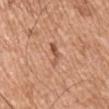Approximately 3 mm at its widest.
This image is a 15 mm lesion crop taken from a total-body photograph.
The patient is a male aged around 55.
On the chest.